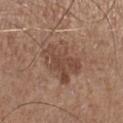<tbp_lesion>
  <biopsy_status>not biopsied; imaged during a skin examination</biopsy_status>
  <lighting>white-light</lighting>
  <lesion_size>
    <long_diameter_mm_approx>5.0</long_diameter_mm_approx>
  </lesion_size>
  <patient>
    <sex>male</sex>
    <age_approx>75</age_approx>
  </patient>
  <image>
    <source>total-body photography crop</source>
    <field_of_view_mm>15</field_of_view_mm>
  </image>
  <site>right lower leg</site>
  <automated_metrics>
    <cielab_L>46</cielab_L>
    <cielab_a>19</cielab_a>
    <cielab_b>27</cielab_b>
    <vs_skin_darker_L>9.0</vs_skin_darker_L>
    <vs_skin_contrast_norm>7.0</vs_skin_contrast_norm>
    <color_variation_0_10>4.0</color_variation_0_10>
    <peripheral_color_asymmetry>1.5</peripheral_color_asymmetry>
    <nevus_likeness_0_100>25</nevus_likeness_0_100>
    <lesion_detection_confidence_0_100>100</lesion_detection_confidence_0_100>
  </automated_metrics>
</tbp_lesion>